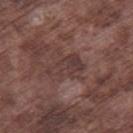follow-up=no biopsy performed (imaged during a skin exam) | subject=male, in their mid- to late 70s | tile lighting=white-light | lesion diameter=about 4.5 mm | image-analysis metrics=a footprint of about 7.5 mm² and a shape eccentricity near 0.85; a lesion–skin lightness drop of about 7 and a normalized lesion–skin contrast near 6; a lesion-detection confidence of about 65/100 | imaging modality=~15 mm tile from a whole-body skin photo | site=the right thigh.